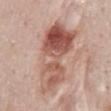Imaged with white-light lighting. A female subject, roughly 50 years of age. Longest diameter approximately 9 mm. The lesion is on the mid back. Cropped from a whole-body photographic skin survey; the tile spans about 15 mm.